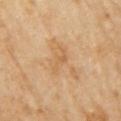biopsy status — total-body-photography surveillance lesion; no biopsy | anatomic site — the right upper arm | tile lighting — cross-polarized illumination | patient — female, in their 70s | lesion diameter — ~3.5 mm (longest diameter) | image source — ~15 mm crop, total-body skin-cancer survey.